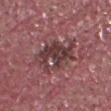Case summary:
– follow-up: catalogued during a skin exam; not biopsied
– site: the head or neck
– patient: male, aged 38–42
– tile lighting: white-light illumination
– size: ≈5.5 mm
– imaging modality: ~15 mm crop, total-body skin-cancer survey